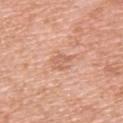| key | value |
|---|---|
| follow-up | total-body-photography surveillance lesion; no biopsy |
| image | total-body-photography crop, ~15 mm field of view |
| subject | female, aged 38–42 |
| anatomic site | the upper back |